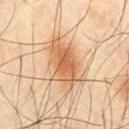Q: Was this lesion biopsied?
A: catalogued during a skin exam; not biopsied
Q: Lesion size?
A: ~7 mm (longest diameter)
Q: Where on the body is the lesion?
A: the front of the torso
Q: What kind of image is this?
A: total-body-photography crop, ~15 mm field of view
Q: What are the patient's age and sex?
A: male, aged approximately 45